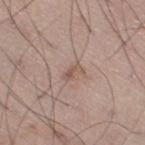A male patient about 60 years old.
A lesion tile, about 15 mm wide, cut from a 3D total-body photograph.
On the leg.